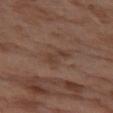Recorded during total-body skin imaging; not selected for excision or biopsy. Cropped from a whole-body photographic skin survey; the tile spans about 15 mm. A female patient, in their mid-50s. On the leg. Approximately 3 mm at its widest.